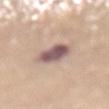- notes — imaged on a skin check; not biopsied
- lesion size — ≈4 mm
- subject — female, aged around 65
- tile lighting — white-light illumination
- acquisition — ~15 mm tile from a whole-body skin photo
- automated metrics — a border-irregularity index near 2/10 and a peripheral color-asymmetry measure near 2; an automated nevus-likeness rating near 15 out of 100 and a lesion-detection confidence of about 100/100
- anatomic site — the lower back